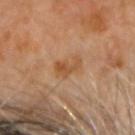Q: Is there a histopathology result?
A: total-body-photography surveillance lesion; no biopsy
Q: How was the tile lit?
A: cross-polarized
Q: What is the imaging modality?
A: 15 mm crop, total-body photography
Q: Patient demographics?
A: male, in their 60s
Q: Lesion location?
A: the head or neck